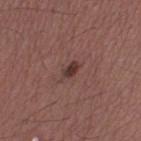notes: imaged on a skin check; not biopsied
anatomic site: the left thigh
patient: male, aged around 40
image source: ~15 mm crop, total-body skin-cancer survey